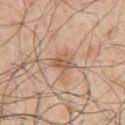Clinical impression: The lesion was photographed on a routine skin check and not biopsied; there is no pathology result. Image and clinical context: A 15 mm close-up extracted from a 3D total-body photography capture. Approximately 2.5 mm at its widest. Imaged with white-light lighting. Located on the left upper arm. A male subject, aged around 70.